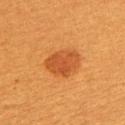<lesion>
<biopsy_status>not biopsied; imaged during a skin examination</biopsy_status>
<site>upper back</site>
<patient>
  <sex>female</sex>
  <age_approx>55</age_approx>
</patient>
<automated_metrics>
  <cielab_L>45</cielab_L>
  <cielab_a>28</cielab_a>
  <cielab_b>41</cielab_b>
  <vs_skin_darker_L>9.0</vs_skin_darker_L>
  <border_irregularity_0_10>2.0</border_irregularity_0_10>
  <color_variation_0_10>2.5</color_variation_0_10>
  <peripheral_color_asymmetry>1.0</peripheral_color_asymmetry>
  <nevus_likeness_0_100>100</nevus_likeness_0_100>
</automated_metrics>
<lesion_size>
  <long_diameter_mm_approx>4.5</long_diameter_mm_approx>
</lesion_size>
<lighting>cross-polarized</lighting>
<image>
  <source>total-body photography crop</source>
  <field_of_view_mm>15</field_of_view_mm>
</image>
</lesion>